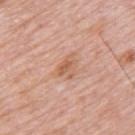The lesion was tiled from a total-body skin photograph and was not biopsied. Captured under white-light illumination. The subject is a male approximately 80 years of age. The lesion is located on the upper back. Cropped from a whole-body photographic skin survey; the tile spans about 15 mm.